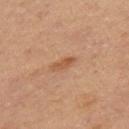Part of a total-body skin-imaging series; this lesion was reviewed on a skin check and was not flagged for biopsy.
The patient is a female aged around 55.
On the right thigh.
This image is a 15 mm lesion crop taken from a total-body photograph.